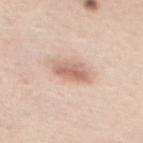Case summary:
- biopsy status: total-body-photography surveillance lesion; no biopsy
- acquisition: ~15 mm crop, total-body skin-cancer survey
- anatomic site: the upper back
- patient: female, about 45 years old